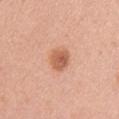Q: Was a biopsy performed?
A: total-body-photography surveillance lesion; no biopsy
Q: What is the lesion's diameter?
A: ~3 mm (longest diameter)
Q: What kind of image is this?
A: ~15 mm tile from a whole-body skin photo
Q: Lesion location?
A: the right upper arm
Q: Illumination type?
A: white-light
Q: Automated lesion metrics?
A: a mean CIELAB color near L≈59 a*≈25 b*≈34, a lesion–skin lightness drop of about 12, and a normalized lesion–skin contrast near 8; a nevus-likeness score of about 95/100 and a lesion-detection confidence of about 100/100
Q: Who is the patient?
A: female, roughly 35 years of age